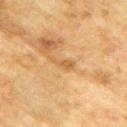Clinical impression: Recorded during total-body skin imaging; not selected for excision or biopsy. Background: On the upper back. A close-up tile cropped from a whole-body skin photograph, about 15 mm across. Imaged with cross-polarized lighting. The subject is a male aged approximately 75. The lesion's longest dimension is about 3.5 mm.